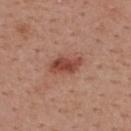The lesion was photographed on a routine skin check and not biopsied; there is no pathology result.
The subject is a male in their mid-50s.
This is a white-light tile.
Automated tile analysis of the lesion measured a mean CIELAB color near L≈46 a*≈26 b*≈28, a lesion–skin lightness drop of about 12, and a lesion-to-skin contrast of about 8.5 (normalized; higher = more distinct). It also reported a nevus-likeness score of about 85/100 and a lesion-detection confidence of about 100/100.
On the upper back.
Cropped from a whole-body photographic skin survey; the tile spans about 15 mm.
About 4 mm across.